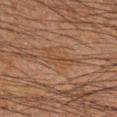image source: ~15 mm tile from a whole-body skin photo | patient: male, aged 33–37 | illumination: cross-polarized illumination | site: the left forearm.